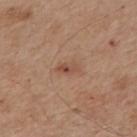workup: total-body-photography surveillance lesion; no biopsy
illumination: white-light
subject: male, about 65 years old
anatomic site: the back
image-analysis metrics: a lesion color around L≈50 a*≈21 b*≈29 in CIELAB, a lesion–skin lightness drop of about 8, and a lesion-to-skin contrast of about 6 (normalized; higher = more distinct); an automated nevus-likeness rating near 20 out of 100 and lesion-presence confidence of about 100/100
acquisition: ~15 mm crop, total-body skin-cancer survey